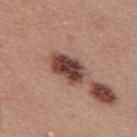Imaged during a routine full-body skin examination; the lesion was not biopsied and no histopathology is available.
A male patient aged around 40.
A close-up tile cropped from a whole-body skin photograph, about 15 mm across.
The lesion's longest dimension is about 4.5 mm.
Located on the upper back.
This is a white-light tile.